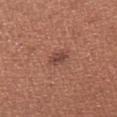Findings:
* biopsy status — no biopsy performed (imaged during a skin exam)
* lesion size — ≈3 mm
* illumination — white-light illumination
* acquisition — 15 mm crop, total-body photography
* subject — female, about 35 years old
* body site — the arm